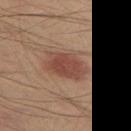Recorded during total-body skin imaging; not selected for excision or biopsy.
The lesion is located on the left thigh.
A male subject roughly 40 years of age.
This is a cross-polarized tile.
A close-up tile cropped from a whole-body skin photograph, about 15 mm across.
Measured at roughly 5 mm in maximum diameter.